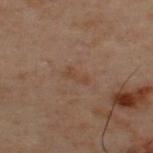Impression:
Captured during whole-body skin photography for melanoma surveillance; the lesion was not biopsied.
Context:
From the back. A male patient, aged 48 to 52. Cropped from a total-body skin-imaging series; the visible field is about 15 mm.